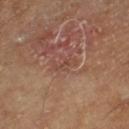Q: Was this lesion biopsied?
A: no biopsy performed (imaged during a skin exam)
Q: Where on the body is the lesion?
A: the right lower leg
Q: What is the lesion's diameter?
A: ≈2.5 mm
Q: Who is the patient?
A: male, approximately 65 years of age
Q: What is the imaging modality?
A: 15 mm crop, total-body photography
Q: Illumination type?
A: cross-polarized illumination